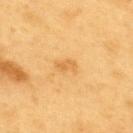follow-up = no biopsy performed (imaged during a skin exam)
site = the upper back
image = 15 mm crop, total-body photography
automated metrics = a footprint of about 3.5 mm² and an eccentricity of roughly 0.85; a lesion color around L≈66 a*≈22 b*≈49 in CIELAB, roughly 8 lightness units darker than nearby skin, and a normalized border contrast of about 5; a nevus-likeness score of about 0/100 and a detector confidence of about 100 out of 100 that the crop contains a lesion
patient = male, about 60 years old
tile lighting = cross-polarized illumination